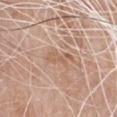– follow-up — total-body-photography surveillance lesion; no biopsy
– subject — male, about 80 years old
– illumination — white-light illumination
– site — the front of the torso
– image — ~15 mm crop, total-body skin-cancer survey